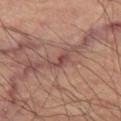| key | value |
|---|---|
| biopsy status | no biopsy performed (imaged during a skin exam) |
| subject | male, aged 63–67 |
| location | the left thigh |
| image source | total-body-photography crop, ~15 mm field of view |